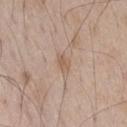notes: imaged on a skin check; not biopsied | automated lesion analysis: a mean CIELAB color near L≈58 a*≈16 b*≈28, about 7 CIELAB-L* units darker than the surrounding skin, and a normalized border contrast of about 5.5; a border-irregularity index near 2.5/10 and radial color variation of about 0.5 | anatomic site: the front of the torso | subject: male, in their 50s | lighting: white-light illumination | image source: 15 mm crop, total-body photography.